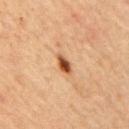Impression: Recorded during total-body skin imaging; not selected for excision or biopsy. Background: Imaged with cross-polarized lighting. A male patient, in their mid-60s. Approximately 2.5 mm at its widest. Cropped from a total-body skin-imaging series; the visible field is about 15 mm. The lesion is on the mid back.